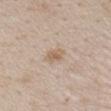workup=no biopsy performed (imaged during a skin exam) | subject=female, aged around 50 | imaging modality=15 mm crop, total-body photography | lesion size=≈2.5 mm | lighting=white-light | site=the back.